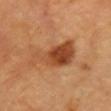Imaged during a routine full-body skin examination; the lesion was not biopsied and no histopathology is available.
A 15 mm crop from a total-body photograph taken for skin-cancer surveillance.
A patient aged 68–72.
From the front of the torso.
The total-body-photography lesion software estimated an area of roughly 16 mm² and a shape eccentricity near 0.9. It also reported roughly 12 lightness units darker than nearby skin and a normalized border contrast of about 8.5. The analysis additionally found a color-variation rating of about 8.5/10 and a peripheral color-asymmetry measure near 3. And it measured an automated nevus-likeness rating near 100 out of 100 and a detector confidence of about 100 out of 100 that the crop contains a lesion.
Captured under cross-polarized illumination.
Measured at roughly 7 mm in maximum diameter.